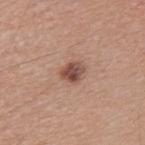Findings:
* workup · no biopsy performed (imaged during a skin exam)
* site · the back
* TBP lesion metrics · a footprint of about 5 mm², a shape eccentricity near 0.6, and a shape-asymmetry score of about 0.25 (0 = symmetric); a border-irregularity rating of about 2/10 and a color-variation rating of about 4.5/10
* patient · male, roughly 40 years of age
* size · ~3 mm (longest diameter)
* tile lighting · white-light illumination
* image source · total-body-photography crop, ~15 mm field of view